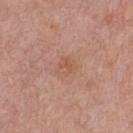{
  "biopsy_status": "not biopsied; imaged during a skin examination",
  "patient": {
    "sex": "male",
    "age_approx": 55
  },
  "site": "front of the torso",
  "image": {
    "source": "total-body photography crop",
    "field_of_view_mm": 15
  },
  "lighting": "white-light",
  "lesion_size": {
    "long_diameter_mm_approx": 2.5
  }
}